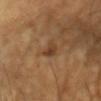workup=total-body-photography surveillance lesion; no biopsy | acquisition=~15 mm tile from a whole-body skin photo | patient=female, approximately 60 years of age | diameter=~2.5 mm (longest diameter) | lighting=cross-polarized illumination | anatomic site=the head or neck.